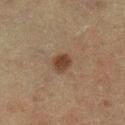The lesion was tiled from a total-body skin photograph and was not biopsied.
On the left lower leg.
A close-up tile cropped from a whole-body skin photograph, about 15 mm across.
Longest diameter approximately 2.5 mm.
Automated image analysis of the tile measured a symmetry-axis asymmetry near 0.2. And it measured an average lesion color of about L≈31 a*≈14 b*≈23 (CIELAB), about 9 CIELAB-L* units darker than the surrounding skin, and a lesion-to-skin contrast of about 9 (normalized; higher = more distinct). The analysis additionally found border irregularity of about 1.5 on a 0–10 scale, internal color variation of about 3 on a 0–10 scale, and a peripheral color-asymmetry measure near 1. The analysis additionally found lesion-presence confidence of about 100/100.
The subject is a male approximately 60 years of age.
The tile uses cross-polarized illumination.